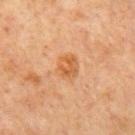The lesion is on the mid back. The recorded lesion diameter is about 3.5 mm. A region of skin cropped from a whole-body photographic capture, roughly 15 mm wide. This is a cross-polarized tile. A male patient, roughly 65 years of age. The lesion-visualizer software estimated an average lesion color of about L≈52 a*≈22 b*≈37 (CIELAB) and a lesion–skin lightness drop of about 7. It also reported a nevus-likeness score of about 70/100 and a detector confidence of about 100 out of 100 that the crop contains a lesion.